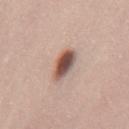workup: no biopsy performed (imaged during a skin exam); acquisition: total-body-photography crop, ~15 mm field of view; site: the mid back; patient: male, aged approximately 45.